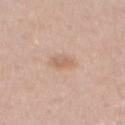biopsy_status: not biopsied; imaged during a skin examination
patient:
  sex: female
  age_approx: 40
lighting: white-light
image:
  source: total-body photography crop
  field_of_view_mm: 15
site: mid back
automated_metrics:
  cielab_L: 62
  cielab_a: 19
  cielab_b: 30
  vs_skin_darker_L: 8.0
  vs_skin_contrast_norm: 5.5
  color_variation_0_10: 1.5
  peripheral_color_asymmetry: 0.5